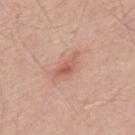No biopsy was performed on this lesion — it was imaged during a full skin examination and was not determined to be concerning. A male subject aged 63 to 67. Imaged with white-light lighting. About 3.5 mm across. On the mid back. A lesion tile, about 15 mm wide, cut from a 3D total-body photograph.